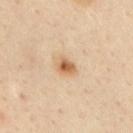No biopsy was performed on this lesion — it was imaged during a full skin examination and was not determined to be concerning.
This is a cross-polarized tile.
On the back.
Cropped from a whole-body photographic skin survey; the tile spans about 15 mm.
A male patient, in their mid- to late 40s.
An algorithmic analysis of the crop reported a mean CIELAB color near L≈60 a*≈19 b*≈36, about 12 CIELAB-L* units darker than the surrounding skin, and a lesion-to-skin contrast of about 8.5 (normalized; higher = more distinct). It also reported a border-irregularity rating of about 2/10.